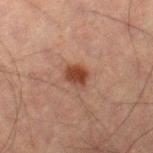Q: Was this lesion biopsied?
A: no biopsy performed (imaged during a skin exam)
Q: How was this image acquired?
A: total-body-photography crop, ~15 mm field of view
Q: What is the anatomic site?
A: the leg
Q: What did automated image analysis measure?
A: a lesion color around L≈32 a*≈19 b*≈24 in CIELAB and a normalized lesion–skin contrast near 9.5; an automated nevus-likeness rating near 95 out of 100
Q: How was the tile lit?
A: cross-polarized illumination
Q: Who is the patient?
A: male, in their 60s